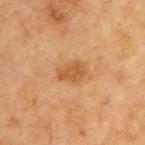Recorded during total-body skin imaging; not selected for excision or biopsy.
A 15 mm close-up tile from a total-body photography series done for melanoma screening.
Captured under cross-polarized illumination.
A male patient, roughly 65 years of age.
About 3 mm across.
The lesion is on the chest.